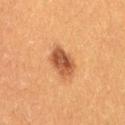Clinical summary:
The recorded lesion diameter is about 4 mm. A female patient, aged 38 to 42. The lesion is located on the right thigh. A 15 mm close-up extracted from a 3D total-body photography capture. An algorithmic analysis of the crop reported a border-irregularity index near 2/10, a color-variation rating of about 4.5/10, and a peripheral color-asymmetry measure near 1.5. And it measured a lesion-detection confidence of about 100/100. The tile uses cross-polarized illumination.